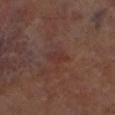Part of a total-body skin-imaging series; this lesion was reviewed on a skin check and was not flagged for biopsy.
The lesion is on the leg.
The tile uses cross-polarized illumination.
Cropped from a total-body skin-imaging series; the visible field is about 15 mm.